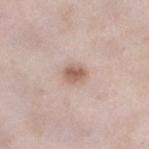Findings:
• follow-up · imaged on a skin check; not biopsied
• lesion size · ≈2.5 mm
• imaging modality · ~15 mm crop, total-body skin-cancer survey
• automated metrics · a within-lesion color-variation index near 3/10 and peripheral color asymmetry of about 1; a detector confidence of about 100 out of 100 that the crop contains a lesion
• subject · female, approximately 30 years of age
• body site · the left lower leg